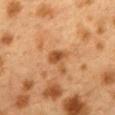Captured during whole-body skin photography for melanoma surveillance; the lesion was not biopsied. A close-up tile cropped from a whole-body skin photograph, about 15 mm across. The lesion-visualizer software estimated an area of roughly 3.5 mm², a shape eccentricity near 0.75, and two-axis asymmetry of about 0.25. It also reported a lesion-to-skin contrast of about 8.5 (normalized; higher = more distinct). And it measured border irregularity of about 2.5 on a 0–10 scale, internal color variation of about 2 on a 0–10 scale, and a peripheral color-asymmetry measure near 0.5. The patient is a female aged 38–42. This is a cross-polarized tile. From the mid back.